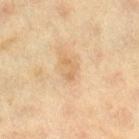follow-up=total-body-photography surveillance lesion; no biopsy
site=the leg
image=total-body-photography crop, ~15 mm field of view
subject=female, about 40 years old
illumination=cross-polarized
diameter=~2.5 mm (longest diameter)
automated metrics=a mean CIELAB color near L≈57 a*≈16 b*≈34 and a normalized lesion–skin contrast near 5.5; an automated nevus-likeness rating near 0 out of 100 and a lesion-detection confidence of about 100/100